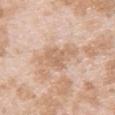imaging modality — total-body-photography crop, ~15 mm field of view | lesion size — ~5.5 mm (longest diameter) | location — the upper back | subject — female, roughly 25 years of age | lighting — white-light.